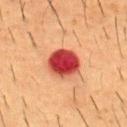Assessment:
Recorded during total-body skin imaging; not selected for excision or biopsy.
Background:
A male subject approximately 55 years of age. Longest diameter approximately 4 mm. Automated image analysis of the tile measured an area of roughly 12 mm², an eccentricity of roughly 0.3, and a shape-asymmetry score of about 0.15 (0 = symmetric). And it measured roughly 20 lightness units darker than nearby skin and a normalized lesion–skin contrast near 14.5. This image is a 15 mm lesion crop taken from a total-body photograph. The lesion is on the chest.